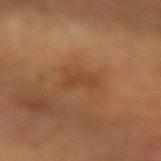| field | value |
|---|---|
| patient | female, aged around 55 |
| image | ~15 mm tile from a whole-body skin photo |
| automated lesion analysis | a lesion area of about 4 mm², an eccentricity of roughly 0.85, and two-axis asymmetry of about 0.45; an average lesion color of about L≈45 a*≈24 b*≈37 (CIELAB), about 6 CIELAB-L* units darker than the surrounding skin, and a normalized border contrast of about 5; a nevus-likeness score of about 0/100 and a detector confidence of about 100 out of 100 that the crop contains a lesion |
| lesion size | ≈3 mm |
| anatomic site | the right forearm |